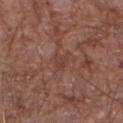| field | value |
|---|---|
| subject | male, about 70 years old |
| image source | ~15 mm tile from a whole-body skin photo |
| TBP lesion metrics | a lesion area of about 3.5 mm² and an eccentricity of roughly 0.7 |
| anatomic site | the arm |
| lighting | white-light illumination |
| size | ~2.5 mm (longest diameter) |